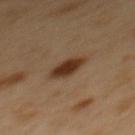Impression:
This lesion was catalogued during total-body skin photography and was not selected for biopsy.